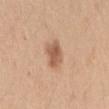size: ≈3 mm; patient: female, about 25 years old; illumination: white-light illumination; site: the right upper arm; acquisition: total-body-photography crop, ~15 mm field of view.